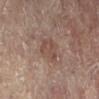Approximately 3 mm at its widest.
On the left leg.
A female patient, aged 78 to 82.
Cropped from a total-body skin-imaging series; the visible field is about 15 mm.
The tile uses cross-polarized illumination.